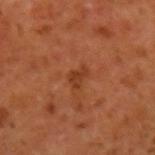Measured at roughly 2.5 mm in maximum diameter.
Cropped from a total-body skin-imaging series; the visible field is about 15 mm.
The lesion is located on the left upper arm.
An algorithmic analysis of the crop reported an automated nevus-likeness rating near 15 out of 100 and a lesion-detection confidence of about 100/100.
A male patient, approximately 60 years of age.